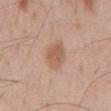biopsy status: total-body-photography surveillance lesion; no biopsy
lesion size: ≈4.5 mm
image: ~15 mm tile from a whole-body skin photo
body site: the mid back
automated lesion analysis: a footprint of about 9 mm² and an outline eccentricity of about 0.75 (0 = round, 1 = elongated)
subject: male, aged around 55